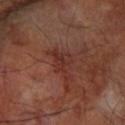follow-up: no biopsy performed (imaged during a skin exam)
image: ~15 mm crop, total-body skin-cancer survey
subject: male, about 65 years old
automated lesion analysis: a lesion color around L≈31 a*≈24 b*≈24 in CIELAB and a lesion–skin lightness drop of about 7; a within-lesion color-variation index near 3.5/10 and a peripheral color-asymmetry measure near 1; a classifier nevus-likeness of about 0/100
anatomic site: the right forearm
lesion size: about 4 mm